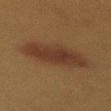Notes:
– follow-up: no biopsy performed (imaged during a skin exam)
– patient: female, aged around 40
– image source: ~15 mm crop, total-body skin-cancer survey
– lighting: cross-polarized
– anatomic site: the mid back
– size: ≈7.5 mm